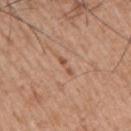Case summary:
- workup: total-body-photography surveillance lesion; no biopsy
- subject: male, about 55 years old
- body site: the right upper arm
- imaging modality: ~15 mm tile from a whole-body skin photo
- automated metrics: an area of roughly 2 mm² and two-axis asymmetry of about 0.4; a lesion color around L≈54 a*≈22 b*≈32 in CIELAB and a lesion-to-skin contrast of about 6 (normalized; higher = more distinct); a nevus-likeness score of about 0/100
- lesion diameter: ≈2.5 mm